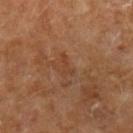{
  "biopsy_status": "not biopsied; imaged during a skin examination",
  "lighting": "cross-polarized",
  "patient": {
    "sex": "male",
    "age_approx": 55
  },
  "lesion_size": {
    "long_diameter_mm_approx": 2.5
  },
  "image": {
    "source": "total-body photography crop",
    "field_of_view_mm": 15
  },
  "site": "left upper arm"
}